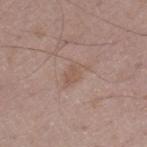Q: Is there a histopathology result?
A: total-body-photography surveillance lesion; no biopsy
Q: Patient demographics?
A: male, approximately 50 years of age
Q: Lesion size?
A: ≈3.5 mm
Q: How was this image acquired?
A: ~15 mm crop, total-body skin-cancer survey
Q: What is the anatomic site?
A: the left thigh
Q: Automated lesion metrics?
A: a shape eccentricity near 0.8; a mean CIELAB color near L≈54 a*≈17 b*≈25, about 6 CIELAB-L* units darker than the surrounding skin, and a normalized border contrast of about 5; a border-irregularity index near 4.5/10, internal color variation of about 1.5 on a 0–10 scale, and a peripheral color-asymmetry measure near 0.5
Q: How was the tile lit?
A: white-light illumination